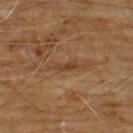{"biopsy_status": "not biopsied; imaged during a skin examination", "lighting": "cross-polarized", "lesion_size": {"long_diameter_mm_approx": 3.0}, "site": "front of the torso", "image": {"source": "total-body photography crop", "field_of_view_mm": 15}, "patient": {"sex": "male", "age_approx": 60}}